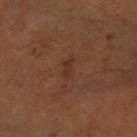Part of a total-body skin-imaging series; this lesion was reviewed on a skin check and was not flagged for biopsy. A close-up tile cropped from a whole-body skin photograph, about 15 mm across. The lesion is located on the left forearm. A male patient aged approximately 65. The tile uses cross-polarized illumination. Measured at roughly 2.5 mm in maximum diameter. Automated tile analysis of the lesion measured a lesion area of about 2.5 mm², a shape eccentricity near 0.9, and two-axis asymmetry of about 0.45. The analysis additionally found an automated nevus-likeness rating near 10 out of 100.